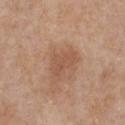Part of a total-body skin-imaging series; this lesion was reviewed on a skin check and was not flagged for biopsy.
A 15 mm close-up extracted from a 3D total-body photography capture.
From the chest.
The lesion's longest dimension is about 4 mm.
A female patient aged 38–42.